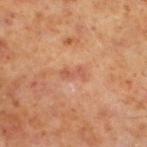{"biopsy_status": "not biopsied; imaged during a skin examination", "patient": {"sex": "male", "age_approx": 60}, "automated_metrics": {"area_mm2_approx": 2.5, "eccentricity": 0.9, "shape_asymmetry": 0.45, "cielab_L": 52, "cielab_a": 25, "cielab_b": 31, "vs_skin_darker_L": 7.0}, "site": "left lower leg", "lighting": "cross-polarized", "lesion_size": {"long_diameter_mm_approx": 2.5}, "image": {"source": "total-body photography crop", "field_of_view_mm": 15}}